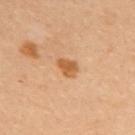The lesion was photographed on a routine skin check and not biopsied; there is no pathology result. The patient is a female roughly 35 years of age. The tile uses cross-polarized illumination. A roughly 15 mm field-of-view crop from a total-body skin photograph. An algorithmic analysis of the crop reported an average lesion color of about L≈57 a*≈23 b*≈40 (CIELAB), roughly 11 lightness units darker than nearby skin, and a normalized border contrast of about 8. Located on the upper back.